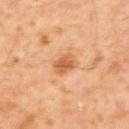No biopsy was performed on this lesion — it was imaged during a full skin examination and was not determined to be concerning. From the upper back. A male subject aged 58 to 62. Imaged with cross-polarized lighting. The total-body-photography lesion software estimated a lesion area of about 4.5 mm² and an eccentricity of roughly 0.85. And it measured a border-irregularity index near 2.5/10, internal color variation of about 2.5 on a 0–10 scale, and peripheral color asymmetry of about 1. Approximately 3 mm at its widest. Cropped from a total-body skin-imaging series; the visible field is about 15 mm.